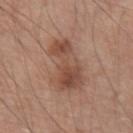Case summary:
* patient — male, roughly 60 years of age
* imaging modality — total-body-photography crop, ~15 mm field of view
* location — the right upper arm